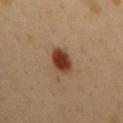Notes:
• workup · catalogued during a skin exam; not biopsied
• lesion size · ≈3.5 mm
• imaging modality · 15 mm crop, total-body photography
• automated lesion analysis · a mean CIELAB color near L≈39 a*≈21 b*≈31; a border-irregularity rating of about 1.5/10 and a peripheral color-asymmetry measure near 1; a classifier nevus-likeness of about 100/100 and a detector confidence of about 100 out of 100 that the crop contains a lesion
• location · the mid back
• lighting · cross-polarized
• subject · male, aged approximately 50